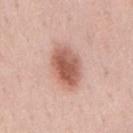Clinical summary:
About 5.5 mm across. A lesion tile, about 15 mm wide, cut from a 3D total-body photograph. A male subject aged 53 to 57. This is a white-light tile. The lesion is located on the mid back.